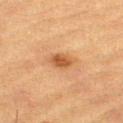illumination = cross-polarized | imaging modality = total-body-photography crop, ~15 mm field of view | subject = male, approximately 85 years of age or older | site = the left thigh | size = ~3.5 mm (longest diameter).